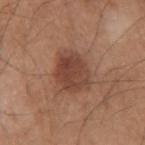• notes: catalogued during a skin exam; not biopsied
• patient: male, roughly 70 years of age
• diameter: ≈5 mm
• image: ~15 mm crop, total-body skin-cancer survey
• anatomic site: the right upper arm
• illumination: white-light illumination